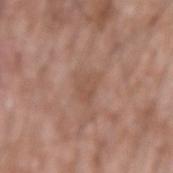Clinical impression: This lesion was catalogued during total-body skin photography and was not selected for biopsy. Background: Cropped from a whole-body photographic skin survey; the tile spans about 15 mm. A male patient, aged 58–62. The lesion is located on the arm. Automated tile analysis of the lesion measured an area of roughly 5 mm², a shape eccentricity near 0.65, and two-axis asymmetry of about 0.35. And it measured a mean CIELAB color near L≈52 a*≈19 b*≈28 and a normalized lesion–skin contrast near 4.5. It also reported a border-irregularity rating of about 3.5/10, internal color variation of about 2 on a 0–10 scale, and peripheral color asymmetry of about 0.5. It also reported lesion-presence confidence of about 100/100.